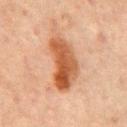The patient is a male approximately 65 years of age. Imaged with cross-polarized lighting. The lesion is on the mid back. A 15 mm crop from a total-body photograph taken for skin-cancer surveillance. Measured at roughly 6.5 mm in maximum diameter. Automated image analysis of the tile measured an average lesion color of about L≈44 a*≈22 b*≈32 (CIELAB), a lesion–skin lightness drop of about 12, and a lesion-to-skin contrast of about 10.5 (normalized; higher = more distinct). And it measured a classifier nevus-likeness of about 85/100 and lesion-presence confidence of about 100/100.